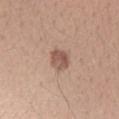| key | value |
|---|---|
| biopsy status | total-body-photography surveillance lesion; no biopsy |
| automated lesion analysis | a color-variation rating of about 3/10 and a peripheral color-asymmetry measure near 1; an automated nevus-likeness rating near 25 out of 100 and lesion-presence confidence of about 100/100 |
| subject | female, aged 23 to 27 |
| image source | 15 mm crop, total-body photography |
| anatomic site | the right forearm |